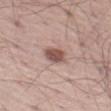A male subject, in their mid- to late 50s.
Captured under white-light illumination.
Measured at roughly 3 mm in maximum diameter.
On the left thigh.
A region of skin cropped from a whole-body photographic capture, roughly 15 mm wide.